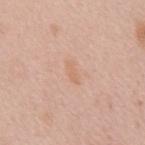| feature | finding |
|---|---|
| biopsy status | imaged on a skin check; not biopsied |
| tile lighting | white-light illumination |
| imaging modality | ~15 mm crop, total-body skin-cancer survey |
| automated lesion analysis | a border-irregularity rating of about 3/10, a within-lesion color-variation index near 0/10, and peripheral color asymmetry of about 0 |
| site | the chest |
| size | ~2.5 mm (longest diameter) |
| subject | male, aged around 55 |